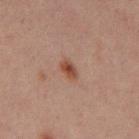Clinical impression: The lesion was photographed on a routine skin check and not biopsied; there is no pathology result. Context: A region of skin cropped from a whole-body photographic capture, roughly 15 mm wide. The lesion is on the mid back. The patient is a male in their 30s. About 2.5 mm across.